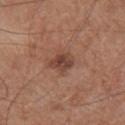diameter: ≈3 mm
lighting: white-light illumination
patient: male, aged approximately 65
image-analysis metrics: an outline eccentricity of about 0.55 (0 = round, 1 = elongated) and a symmetry-axis asymmetry near 0.25; a border-irregularity index near 2.5/10, a within-lesion color-variation index near 5.5/10, and radial color variation of about 2
site: the front of the torso
image: ~15 mm tile from a whole-body skin photo